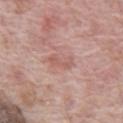This lesion was catalogued during total-body skin photography and was not selected for biopsy.
The patient is a male aged 68–72.
The lesion is located on the abdomen.
The tile uses white-light illumination.
A 15 mm crop from a total-body photograph taken for skin-cancer surveillance.
The lesion-visualizer software estimated a lesion area of about 4.5 mm². It also reported a classifier nevus-likeness of about 0/100 and a lesion-detection confidence of about 100/100.
About 3 mm across.